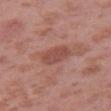| feature | finding |
|---|---|
| workup | catalogued during a skin exam; not biopsied |
| lesion diameter | ≈4 mm |
| anatomic site | the arm |
| patient | male, roughly 40 years of age |
| acquisition | ~15 mm crop, total-body skin-cancer survey |
| illumination | white-light illumination |